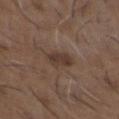Clinical summary:
Located on the chest. The lesion-visualizer software estimated a nevus-likeness score of about 35/100 and lesion-presence confidence of about 100/100. A close-up tile cropped from a whole-body skin photograph, about 15 mm across. Approximately 3 mm at its widest. Imaged with white-light lighting. The patient is a male approximately 50 years of age.